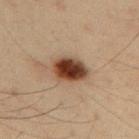biopsy status: imaged on a skin check; not biopsied
site: the right upper arm
automated metrics: a lesion area of about 11 mm², a shape eccentricity near 0.75, and a symmetry-axis asymmetry near 0.15; border irregularity of about 1.5 on a 0–10 scale and radial color variation of about 2; a nevus-likeness score of about 100/100
image: ~15 mm crop, total-body skin-cancer survey
size: ~4.5 mm (longest diameter)
subject: male, roughly 35 years of age